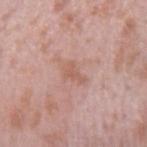This lesion was catalogued during total-body skin photography and was not selected for biopsy. This is a white-light tile. The patient is a male aged 38–42. The lesion is located on the right upper arm. A roughly 15 mm field-of-view crop from a total-body skin photograph.